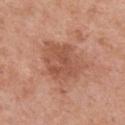workup = total-body-photography surveillance lesion; no biopsy | image-analysis metrics = an average lesion color of about L≈53 a*≈24 b*≈31 (CIELAB) and about 9 CIELAB-L* units darker than the surrounding skin; an automated nevus-likeness rating near 5 out of 100 and lesion-presence confidence of about 100/100 | patient = female, about 50 years old | lighting = white-light illumination | acquisition = ~15 mm tile from a whole-body skin photo | body site = the chest | size = ≈6 mm.